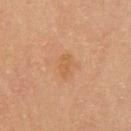| feature | finding |
|---|---|
| follow-up | total-body-photography surveillance lesion; no biopsy |
| acquisition | ~15 mm tile from a whole-body skin photo |
| patient | male, aged 58–62 |
| tile lighting | cross-polarized illumination |